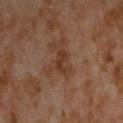  biopsy_status: not biopsied; imaged during a skin examination
  image:
    source: total-body photography crop
    field_of_view_mm: 15
  site: chest
  patient:
    sex: male
    age_approx: 60